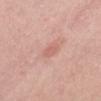Assessment: Part of a total-body skin-imaging series; this lesion was reviewed on a skin check and was not flagged for biopsy. Context: Measured at roughly 2.5 mm in maximum diameter. A female patient, in their 60s. A 15 mm close-up extracted from a 3D total-body photography capture. The lesion-visualizer software estimated a footprint of about 3.5 mm², an outline eccentricity of about 0.8 (0 = round, 1 = elongated), and a shape-asymmetry score of about 0.3 (0 = symmetric). It also reported a border-irregularity index near 2.5/10, a within-lesion color-variation index near 2.5/10, and peripheral color asymmetry of about 1. The lesion is on the abdomen.